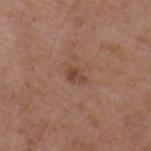Recorded during total-body skin imaging; not selected for excision or biopsy. A 15 mm close-up extracted from a 3D total-body photography capture. A male subject aged 48 to 52. The lesion's longest dimension is about 2.5 mm. The lesion is located on the right upper arm.